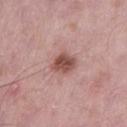| field | value |
|---|---|
| biopsy status | imaged on a skin check; not biopsied |
| subject | male, aged 53 to 57 |
| body site | the left thigh |
| acquisition | ~15 mm tile from a whole-body skin photo |